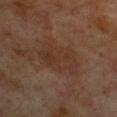notes=imaged on a skin check; not biopsied
location=the front of the torso
image source=~15 mm crop, total-body skin-cancer survey
patient=female, aged around 60